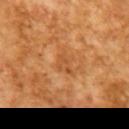biopsy_status: not biopsied; imaged during a skin examination
lesion_size:
  long_diameter_mm_approx: 2.5
lighting: cross-polarized
automated_metrics:
  area_mm2_approx: 3.5
  eccentricity: 0.75
  nevus_likeness_0_100: 0
  lesion_detection_confidence_0_100: 100
image:
  source: total-body photography crop
  field_of_view_mm: 15
patient:
  sex: male
  age_approx: 65